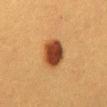<tbp_lesion>
<biopsy_status>not biopsied; imaged during a skin examination</biopsy_status>
<image>
  <source>total-body photography crop</source>
  <field_of_view_mm>15</field_of_view_mm>
</image>
<lighting>cross-polarized</lighting>
<site>abdomen</site>
<automated_metrics>
  <area_mm2_approx>11.0</area_mm2_approx>
  <eccentricity>0.55</eccentricity>
  <shape_asymmetry>0.15</shape_asymmetry>
  <cielab_L>37</cielab_L>
  <cielab_a>22</cielab_a>
  <cielab_b>32</cielab_b>
  <vs_skin_darker_L>16.0</vs_skin_darker_L>
  <vs_skin_contrast_norm>13.0</vs_skin_contrast_norm>
</automated_metrics>
<patient>
  <sex>female</sex>
  <age_approx>40</age_approx>
</patient>
<lesion_size>
  <long_diameter_mm_approx>4.0</long_diameter_mm_approx>
</lesion_size>
</tbp_lesion>